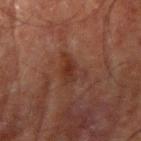This lesion was catalogued during total-body skin photography and was not selected for biopsy.
About 4 mm across.
Imaged with cross-polarized lighting.
A male patient, aged 68–72.
An algorithmic analysis of the crop reported a footprint of about 6.5 mm², an outline eccentricity of about 0.75 (0 = round, 1 = elongated), and two-axis asymmetry of about 0.5. The software also gave a lesion color around L≈27 a*≈19 b*≈23 in CIELAB, roughly 6 lightness units darker than nearby skin, and a normalized border contrast of about 6.5. It also reported a classifier nevus-likeness of about 0/100 and lesion-presence confidence of about 100/100.
A region of skin cropped from a whole-body photographic capture, roughly 15 mm wide.
From the right upper arm.